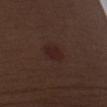notes=no biopsy performed (imaged during a skin exam) | illumination=white-light illumination | automated lesion analysis=an average lesion color of about L≈22 a*≈18 b*≈18 (CIELAB) and roughly 5 lightness units darker than nearby skin | acquisition=~15 mm crop, total-body skin-cancer survey | body site=the right upper arm | subject=male, aged around 70.